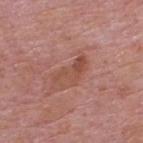Notes:
* follow-up — imaged on a skin check; not biopsied
* lesion size — about 4.5 mm
* anatomic site — the upper back
* subject — male, aged around 75
* automated metrics — an average lesion color of about L≈49 a*≈24 b*≈28 (CIELAB) and a lesion-to-skin contrast of about 6.5 (normalized; higher = more distinct); an automated nevus-likeness rating near 0 out of 100
* tile lighting — white-light
* image source — 15 mm crop, total-body photography